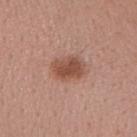<case>
  <biopsy_status>not biopsied; imaged during a skin examination</biopsy_status>
  <patient>
    <sex>female</sex>
    <age_approx>30</age_approx>
  </patient>
  <image>
    <source>total-body photography crop</source>
    <field_of_view_mm>15</field_of_view_mm>
  </image>
  <site>left forearm</site>
</case>